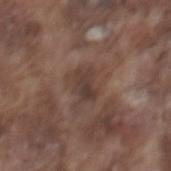Q: Was this lesion biopsied?
A: total-body-photography surveillance lesion; no biopsy
Q: Lesion location?
A: the mid back
Q: Patient demographics?
A: male, roughly 75 years of age
Q: What kind of image is this?
A: ~15 mm crop, total-body skin-cancer survey
Q: Lesion size?
A: ~3 mm (longest diameter)